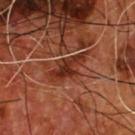workup = imaged on a skin check; not biopsied | imaging modality = 15 mm crop, total-body photography | tile lighting = cross-polarized illumination | patient = male, about 55 years old | anatomic site = the chest | lesion diameter = ~5.5 mm (longest diameter) | TBP lesion metrics = an eccentricity of roughly 0.9; a lesion color around L≈29 a*≈25 b*≈29 in CIELAB and a normalized lesion–skin contrast near 9; border irregularity of about 5 on a 0–10 scale, a color-variation rating of about 4/10, and a peripheral color-asymmetry measure near 1; an automated nevus-likeness rating near 0 out of 100 and a detector confidence of about 100 out of 100 that the crop contains a lesion.